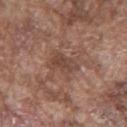Captured during whole-body skin photography for melanoma surveillance; the lesion was not biopsied. A male subject in their mid- to late 70s. The recorded lesion diameter is about 3 mm. This is a white-light tile. A 15 mm close-up extracted from a 3D total-body photography capture. The lesion is located on the chest.